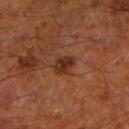image = 15 mm crop, total-body photography | patient = male, in their mid-60s | anatomic site = the left lower leg | lesion diameter = about 2.5 mm | illumination = cross-polarized illumination.